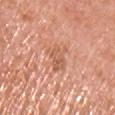| key | value |
|---|---|
| follow-up | no biopsy performed (imaged during a skin exam) |
| illumination | white-light |
| size | ~4 mm (longest diameter) |
| acquisition | ~15 mm tile from a whole-body skin photo |
| patient | male, aged 68 to 72 |
| anatomic site | the chest |